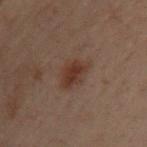Imaged during a routine full-body skin examination; the lesion was not biopsied and no histopathology is available. Located on the upper back. Imaged with cross-polarized lighting. A roughly 15 mm field-of-view crop from a total-body skin photograph. Automated tile analysis of the lesion measured an outline eccentricity of about 0.7 (0 = round, 1 = elongated) and a symmetry-axis asymmetry near 0.25. And it measured a border-irregularity rating of about 3/10, a within-lesion color-variation index near 3/10, and peripheral color asymmetry of about 1. A male patient aged around 40. The recorded lesion diameter is about 4 mm.